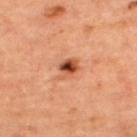{
  "biopsy_status": "not biopsied; imaged during a skin examination",
  "image": {
    "source": "total-body photography crop",
    "field_of_view_mm": 15
  },
  "patient": {
    "sex": "female",
    "age_approx": 45
  },
  "lesion_size": {
    "long_diameter_mm_approx": 3.0
  },
  "automated_metrics": {
    "cielab_L": 50,
    "cielab_a": 28,
    "cielab_b": 36,
    "vs_skin_darker_L": 15.0,
    "vs_skin_contrast_norm": 10.0
  },
  "site": "upper back"
}